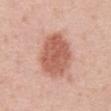Automated image analysis of the tile measured a lesion area of about 20 mm², an eccentricity of roughly 0.7, and two-axis asymmetry of about 0.1. And it measured a mean CIELAB color near L≈59 a*≈25 b*≈29, about 13 CIELAB-L* units darker than the surrounding skin, and a lesion-to-skin contrast of about 8.5 (normalized; higher = more distinct). The tile uses white-light illumination. On the abdomen. This image is a 15 mm lesion crop taken from a total-body photograph. The patient is a male aged approximately 55.